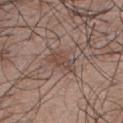Impression:
Imaged during a routine full-body skin examination; the lesion was not biopsied and no histopathology is available.
Image and clinical context:
Approximately 4.5 mm at its widest. Captured under white-light illumination. Cropped from a whole-body photographic skin survey; the tile spans about 15 mm. A male subject in their 50s. The lesion is located on the back. Automated image analysis of the tile measured a footprint of about 7.5 mm² and a shape eccentricity near 0.85. The analysis additionally found a lesion color around L≈47 a*≈16 b*≈24 in CIELAB, a lesion–skin lightness drop of about 7, and a lesion-to-skin contrast of about 5.5 (normalized; higher = more distinct). It also reported a classifier nevus-likeness of about 0/100 and lesion-presence confidence of about 100/100.